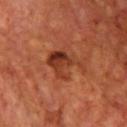Assessment: No biopsy was performed on this lesion — it was imaged during a full skin examination and was not determined to be concerning. Image and clinical context: From the chest. Automated tile analysis of the lesion measured an area of roughly 10 mm², an outline eccentricity of about 0.65 (0 = round, 1 = elongated), and two-axis asymmetry of about 0.55. The analysis additionally found an average lesion color of about L≈38 a*≈29 b*≈34 (CIELAB), a lesion–skin lightness drop of about 10, and a normalized lesion–skin contrast near 8. And it measured a nevus-likeness score of about 30/100 and a lesion-detection confidence of about 100/100. Measured at roughly 4.5 mm in maximum diameter. The subject is a male aged around 60. A 15 mm close-up tile from a total-body photography series done for melanoma screening. Captured under cross-polarized illumination.